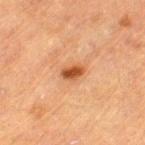This lesion was catalogued during total-body skin photography and was not selected for biopsy. A male subject in their mid- to late 80s or older. Captured under cross-polarized illumination. Cropped from a total-body skin-imaging series; the visible field is about 15 mm. From the leg. An algorithmic analysis of the crop reported a footprint of about 3.5 mm² and a symmetry-axis asymmetry near 0.2. It also reported a border-irregularity index near 2/10 and internal color variation of about 2 on a 0–10 scale. And it measured a lesion-detection confidence of about 100/100. Longest diameter approximately 2.5 mm.